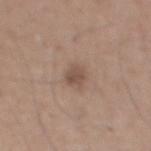Notes:
• biopsy status — total-body-photography surveillance lesion; no biopsy
• illumination — white-light
• image-analysis metrics — a lesion area of about 4 mm² and a shape eccentricity near 0.75; an average lesion color of about L≈50 a*≈16 b*≈24 (CIELAB) and about 9 CIELAB-L* units darker than the surrounding skin; a classifier nevus-likeness of about 20/100 and a detector confidence of about 100 out of 100 that the crop contains a lesion
• imaging modality — 15 mm crop, total-body photography
• location — the chest
• subject — male, aged approximately 65
• size — ≈2.5 mm